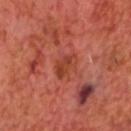Assessment:
Imaged during a routine full-body skin examination; the lesion was not biopsied and no histopathology is available.
Image and clinical context:
Approximately 3 mm at its widest. Captured under cross-polarized illumination. A male subject approximately 65 years of age. A 15 mm close-up extracted from a 3D total-body photography capture.